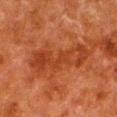Case summary:
• follow-up · no biopsy performed (imaged during a skin exam)
• patient · male, in their 80s
• lesion diameter · about 8.5 mm
• image · ~15 mm crop, total-body skin-cancer survey
• site · the left lower leg